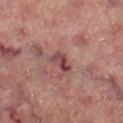biopsy status: total-body-photography surveillance lesion; no biopsy | illumination: cross-polarized illumination | body site: the right lower leg | subject: female, aged around 70 | acquisition: total-body-photography crop, ~15 mm field of view.